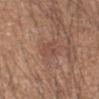No biopsy was performed on this lesion — it was imaged during a full skin examination and was not determined to be concerning. On the right forearm. A male subject approximately 50 years of age. A 15 mm crop from a total-body photograph taken for skin-cancer surveillance.